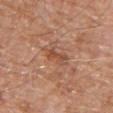• biopsy status · catalogued during a skin exam; not biopsied
• subject · male, aged approximately 80
• lighting · white-light
• image · ~15 mm crop, total-body skin-cancer survey
• lesion diameter · ~3.5 mm (longest diameter)
• anatomic site · the chest